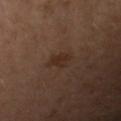<case>
<biopsy_status>not biopsied; imaged during a skin examination</biopsy_status>
<lighting>cross-polarized</lighting>
<lesion_size>
  <long_diameter_mm_approx>2.5</long_diameter_mm_approx>
</lesion_size>
<image>
  <source>total-body photography crop</source>
  <field_of_view_mm>15</field_of_view_mm>
</image>
<site>right forearm</site>
<patient>
  <sex>female</sex>
  <age_approx>60</age_approx>
</patient>
</case>